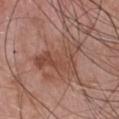<tbp_lesion>
  <biopsy_status>not biopsied; imaged during a skin examination</biopsy_status>
  <image>
    <source>total-body photography crop</source>
    <field_of_view_mm>15</field_of_view_mm>
  </image>
  <automated_metrics>
    <area_mm2_approx>15.0</area_mm2_approx>
    <eccentricity>0.9</eccentricity>
    <shape_asymmetry>0.6</shape_asymmetry>
    <nevus_likeness_0_100>0</nevus_likeness_0_100>
    <lesion_detection_confidence_0_100>100</lesion_detection_confidence_0_100>
  </automated_metrics>
  <site>chest</site>
  <lighting>white-light</lighting>
  <patient>
    <sex>male</sex>
    <age_approx>55</age_approx>
  </patient>
  <lesion_size>
    <long_diameter_mm_approx>7.0</long_diameter_mm_approx>
  </lesion_size>
</tbp_lesion>